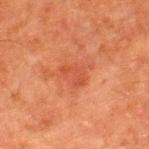| field | value |
|---|---|
| workup | total-body-photography surveillance lesion; no biopsy |
| location | the left thigh |
| diameter | about 2.5 mm |
| subject | male, aged around 80 |
| tile lighting | cross-polarized |
| imaging modality | ~15 mm tile from a whole-body skin photo |